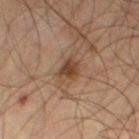The lesion was tiled from a total-body skin photograph and was not biopsied. From the left thigh. This image is a 15 mm lesion crop taken from a total-body photograph. A male subject aged around 55. An algorithmic analysis of the crop reported a footprint of about 5.5 mm², an eccentricity of roughly 0.75, and two-axis asymmetry of about 0.3. The software also gave a lesion color around L≈39 a*≈16 b*≈28 in CIELAB and a normalized lesion–skin contrast near 8. It also reported border irregularity of about 3 on a 0–10 scale, a within-lesion color-variation index near 4/10, and radial color variation of about 1.5.